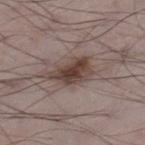follow-up=catalogued during a skin exam; not biopsied
image source=~15 mm crop, total-body skin-cancer survey
subject=male, aged around 70
location=the left thigh